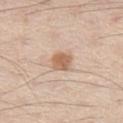Captured during whole-body skin photography for melanoma surveillance; the lesion was not biopsied.
The recorded lesion diameter is about 2.5 mm.
The lesion is located on the left thigh.
A male subject, aged 58 to 62.
The tile uses white-light illumination.
A region of skin cropped from a whole-body photographic capture, roughly 15 mm wide.